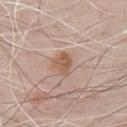This lesion was catalogued during total-body skin photography and was not selected for biopsy. A roughly 15 mm field-of-view crop from a total-body skin photograph. The lesion is on the chest. A male subject, aged approximately 70.